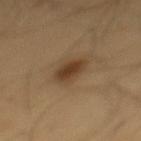| feature | finding |
|---|---|
| biopsy status | catalogued during a skin exam; not biopsied |
| imaging modality | ~15 mm tile from a whole-body skin photo |
| patient | male, roughly 55 years of age |
| site | the mid back |